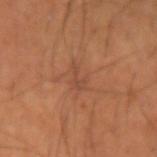Assessment: The lesion was photographed on a routine skin check and not biopsied; there is no pathology result. Image and clinical context: From the left forearm. A male patient, aged around 50. A 15 mm close-up tile from a total-body photography series done for melanoma screening.